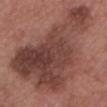<case>
  <biopsy_status>not biopsied; imaged during a skin examination</biopsy_status>
  <site>front of the torso</site>
  <automated_metrics>
    <area_mm2_approx>75.0</area_mm2_approx>
    <eccentricity>0.8</eccentricity>
    <shape_asymmetry>0.55</shape_asymmetry>
    <border_irregularity_0_10>7.0</border_irregularity_0_10>
    <color_variation_0_10>6.5</color_variation_0_10>
    <peripheral_color_asymmetry>2.5</peripheral_color_asymmetry>
  </automated_metrics>
  <image>
    <source>total-body photography crop</source>
    <field_of_view_mm>15</field_of_view_mm>
  </image>
  <patient>
    <sex>male</sex>
    <age_approx>50</age_approx>
  </patient>
  <lesion_size>
    <long_diameter_mm_approx>13.5</long_diameter_mm_approx>
  </lesion_size>
  <lighting>white-light</lighting>
</case>